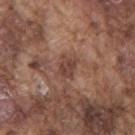Q: Is there a histopathology result?
A: total-body-photography surveillance lesion; no biopsy
Q: Who is the patient?
A: male, in their mid- to late 70s
Q: Lesion size?
A: about 2.5 mm
Q: How was this image acquired?
A: total-body-photography crop, ~15 mm field of view
Q: Where on the body is the lesion?
A: the mid back
Q: Illumination type?
A: white-light
Q: Automated lesion metrics?
A: a footprint of about 4.5 mm² and an outline eccentricity of about 0.6 (0 = round, 1 = elongated); an average lesion color of about L≈42 a*≈21 b*≈25 (CIELAB), roughly 9 lightness units darker than nearby skin, and a lesion-to-skin contrast of about 7.5 (normalized; higher = more distinct); a border-irregularity rating of about 3/10 and radial color variation of about 1; an automated nevus-likeness rating near 5 out of 100